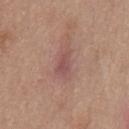Part of a total-body skin-imaging series; this lesion was reviewed on a skin check and was not flagged for biopsy.
About 3.5 mm across.
Captured under white-light illumination.
The patient is a male aged around 55.
Located on the chest.
Cropped from a whole-body photographic skin survey; the tile spans about 15 mm.